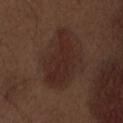Q: Is there a histopathology result?
A: total-body-photography surveillance lesion; no biopsy
Q: Lesion location?
A: the abdomen
Q: What kind of image is this?
A: ~15 mm tile from a whole-body skin photo
Q: Patient demographics?
A: male, aged 68 to 72